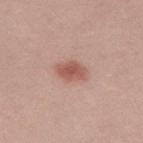This lesion was catalogued during total-body skin photography and was not selected for biopsy.
About 3.5 mm across.
A male patient approximately 30 years of age.
A lesion tile, about 15 mm wide, cut from a 3D total-body photograph.
Captured under white-light illumination.